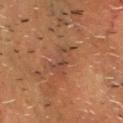Q: Is there a histopathology result?
A: no biopsy performed (imaged during a skin exam)
Q: Where on the body is the lesion?
A: the chest
Q: What is the imaging modality?
A: ~15 mm crop, total-body skin-cancer survey
Q: What is the lesion's diameter?
A: ≈4 mm
Q: What are the patient's age and sex?
A: male, aged 73–77